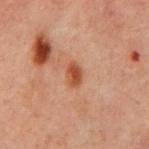workup = catalogued during a skin exam; not biopsied | location = the chest | TBP lesion metrics = a detector confidence of about 100 out of 100 that the crop contains a lesion | image source = 15 mm crop, total-body photography | lesion diameter = ≈2.5 mm | illumination = cross-polarized | subject = male, aged around 60.